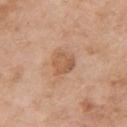No biopsy was performed on this lesion — it was imaged during a full skin examination and was not determined to be concerning. A region of skin cropped from a whole-body photographic capture, roughly 15 mm wide. The total-body-photography lesion software estimated a border-irregularity index near 2.5/10, internal color variation of about 3 on a 0–10 scale, and peripheral color asymmetry of about 1. The analysis additionally found an automated nevus-likeness rating near 5 out of 100. From the right upper arm. Longest diameter approximately 3 mm. Captured under white-light illumination. A female patient aged 73–77.